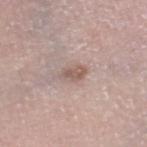Captured during whole-body skin photography for melanoma surveillance; the lesion was not biopsied.
Cropped from a whole-body photographic skin survey; the tile spans about 15 mm.
On the right thigh.
A female patient approximately 55 years of age.
The lesion's longest dimension is about 3 mm.